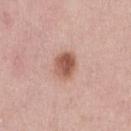follow-up = catalogued during a skin exam; not biopsied
tile lighting = white-light
location = the abdomen
image-analysis metrics = a lesion area of about 7 mm², a shape eccentricity near 0.6, and a symmetry-axis asymmetry near 0.2; a lesion color around L≈56 a*≈23 b*≈28 in CIELAB, roughly 14 lightness units darker than nearby skin, and a normalized lesion–skin contrast near 9; a classifier nevus-likeness of about 100/100 and a detector confidence of about 100 out of 100 that the crop contains a lesion
size = ~3.5 mm (longest diameter)
image source = total-body-photography crop, ~15 mm field of view
patient = female, aged 28–32